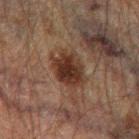This lesion was catalogued during total-body skin photography and was not selected for biopsy.
A roughly 15 mm field-of-view crop from a total-body skin photograph.
Imaged with cross-polarized lighting.
Longest diameter approximately 4.5 mm.
From the left thigh.
A male subject in their 60s.